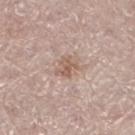workup: catalogued during a skin exam; not biopsied
location: the right leg
image: ~15 mm crop, total-body skin-cancer survey
lesion size: ~2.5 mm (longest diameter)
lighting: white-light illumination
patient: male, aged 68 to 72